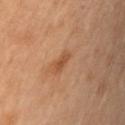Clinical impression: The lesion was photographed on a routine skin check and not biopsied; there is no pathology result. Image and clinical context: A 15 mm close-up tile from a total-body photography series done for melanoma screening. On the left upper arm. This is a cross-polarized tile. An algorithmic analysis of the crop reported a footprint of about 3 mm² and a shape-asymmetry score of about 0.25 (0 = symmetric). A female patient approximately 50 years of age.